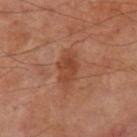Clinical impression:
The lesion was photographed on a routine skin check and not biopsied; there is no pathology result.
Clinical summary:
The lesion is on the right thigh. Imaged with cross-polarized lighting. This image is a 15 mm lesion crop taken from a total-body photograph. Approximately 4 mm at its widest. A male subject aged 68–72.